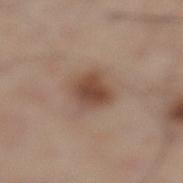Case summary:
* notes — total-body-photography surveillance lesion; no biopsy
* site — the left lower leg
* image source — 15 mm crop, total-body photography
* lesion diameter — ≈4 mm
* illumination — white-light illumination
* subject — male, in their 60s